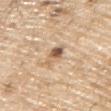biopsy status = no biopsy performed (imaged during a skin exam) | imaging modality = total-body-photography crop, ~15 mm field of view | subject = male, aged 68–72 | size = ~3 mm (longest diameter) | lighting = white-light | site = the right upper arm | automated metrics = a shape eccentricity near 0.75 and two-axis asymmetry of about 0.35; border irregularity of about 3.5 on a 0–10 scale, internal color variation of about 9 on a 0–10 scale, and peripheral color asymmetry of about 4; a nevus-likeness score of about 85/100 and lesion-presence confidence of about 100/100.